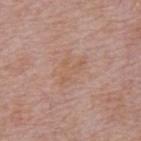Notes:
• image source — ~15 mm crop, total-body skin-cancer survey
• patient — male, aged around 75
• lesion size — about 4 mm
• body site — the mid back
• illumination — white-light illumination